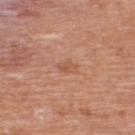Clinical impression: No biopsy was performed on this lesion — it was imaged during a full skin examination and was not determined to be concerning. Acquisition and patient details: The lesion is on the back. The subject is a male approximately 70 years of age. A lesion tile, about 15 mm wide, cut from a 3D total-body photograph.